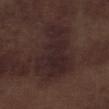| key | value |
|---|---|
| biopsy status | catalogued during a skin exam; not biopsied |
| lesion diameter | about 9 mm |
| location | the left lower leg |
| imaging modality | total-body-photography crop, ~15 mm field of view |
| automated lesion analysis | an average lesion color of about L≈23 a*≈15 b*≈14 (CIELAB), roughly 6 lightness units darker than nearby skin, and a lesion-to-skin contrast of about 8.5 (normalized; higher = more distinct) |
| lighting | white-light illumination |
| subject | male, aged around 70 |